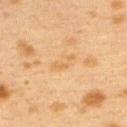{
  "biopsy_status": "not biopsied; imaged during a skin examination",
  "patient": {
    "sex": "female",
    "age_approx": 40
  },
  "image": {
    "source": "total-body photography crop",
    "field_of_view_mm": 15
  },
  "lighting": "cross-polarized",
  "site": "upper back",
  "lesion_size": {
    "long_diameter_mm_approx": 3.0
  }
}